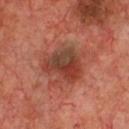biopsy status = imaged on a skin check; not biopsied
anatomic site = the front of the torso
lesion diameter = about 4.5 mm
TBP lesion metrics = a border-irregularity index near 3.5/10, a within-lesion color-variation index near 10/10, and a peripheral color-asymmetry measure near 5
patient = male, approximately 70 years of age
lighting = cross-polarized
image source = 15 mm crop, total-body photography